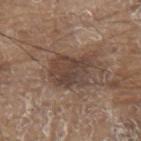No biopsy was performed on this lesion — it was imaged during a full skin examination and was not determined to be concerning. The total-body-photography lesion software estimated an area of roughly 12 mm², an eccentricity of roughly 0.7, and a symmetry-axis asymmetry near 0.3. And it measured an average lesion color of about L≈41 a*≈15 b*≈23 (CIELAB), a lesion–skin lightness drop of about 10, and a lesion-to-skin contrast of about 8 (normalized; higher = more distinct). The software also gave a classifier nevus-likeness of about 5/100. A male patient, approximately 80 years of age. On the mid back. A 15 mm close-up extracted from a 3D total-body photography capture.